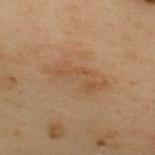Clinical impression: This lesion was catalogued during total-body skin photography and was not selected for biopsy. Image and clinical context: A male subject, roughly 55 years of age. The recorded lesion diameter is about 6.5 mm. Cropped from a total-body skin-imaging series; the visible field is about 15 mm. Located on the upper back. The tile uses cross-polarized illumination.